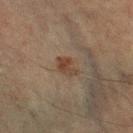Recorded during total-body skin imaging; not selected for excision or biopsy. The lesion is located on the right lower leg. Measured at roughly 3 mm in maximum diameter. A close-up tile cropped from a whole-body skin photograph, about 15 mm across. Captured under cross-polarized illumination. The lesion-visualizer software estimated a footprint of about 4 mm² and an outline eccentricity of about 0.75 (0 = round, 1 = elongated). The software also gave a lesion color around L≈34 a*≈14 b*≈23 in CIELAB and a normalized lesion–skin contrast near 7.5. The analysis additionally found an automated nevus-likeness rating near 60 out of 100 and lesion-presence confidence of about 100/100. The patient is a male aged 58–62.